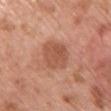On the chest. A 15 mm close-up tile from a total-body photography series done for melanoma screening. This is a white-light tile. Approximately 4 mm at its widest. A female patient, aged around 60.